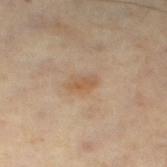Recorded during total-body skin imaging; not selected for excision or biopsy. Cropped from a total-body skin-imaging series; the visible field is about 15 mm. On the left thigh. An algorithmic analysis of the crop reported a border-irregularity index near 2.5/10 and a peripheral color-asymmetry measure near 0.5. It also reported a classifier nevus-likeness of about 30/100. The subject is a male aged 58 to 62. This is a cross-polarized tile.